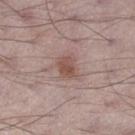Impression:
No biopsy was performed on this lesion — it was imaged during a full skin examination and was not determined to be concerning.
Acquisition and patient details:
A 15 mm close-up extracted from a 3D total-body photography capture. A male subject aged 68–72. Located on the right thigh. Imaged with white-light lighting. Longest diameter approximately 3 mm.